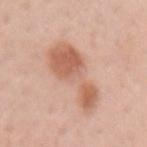Clinical impression:
The lesion was photographed on a routine skin check and not biopsied; there is no pathology result.
Acquisition and patient details:
Imaged with white-light lighting. On the arm. Automated tile analysis of the lesion measured a lesion area of about 20 mm² and a shape-asymmetry score of about 0.45 (0 = symmetric). The software also gave roughly 11 lightness units darker than nearby skin and a lesion-to-skin contrast of about 7.5 (normalized; higher = more distinct). It also reported a nevus-likeness score of about 45/100 and lesion-presence confidence of about 100/100. A female subject, in their mid- to late 40s. A roughly 15 mm field-of-view crop from a total-body skin photograph.